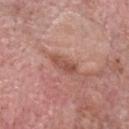follow-up = no biopsy performed (imaged during a skin exam) | body site = the head or neck | illumination = white-light illumination | subject = male, aged approximately 60 | image source = total-body-photography crop, ~15 mm field of view | lesion diameter = ≈3 mm.